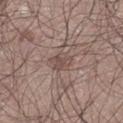{
  "lighting": "white-light",
  "image": {
    "source": "total-body photography crop",
    "field_of_view_mm": 15
  },
  "site": "leg",
  "patient": {
    "sex": "male",
    "age_approx": 65
  }
}